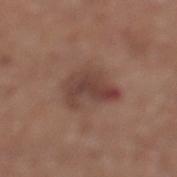The lesion was tiled from a total-body skin photograph and was not biopsied. The lesion is located on the leg. Longest diameter approximately 5 mm. Cropped from a total-body skin-imaging series; the visible field is about 15 mm. A male subject in their mid-60s. The tile uses white-light illumination. Automated tile analysis of the lesion measured an average lesion color of about L≈42 a*≈20 b*≈23 (CIELAB), a lesion–skin lightness drop of about 9, and a lesion-to-skin contrast of about 7.5 (normalized; higher = more distinct).